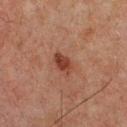Q: Is there a histopathology result?
A: total-body-photography surveillance lesion; no biopsy
Q: What are the patient's age and sex?
A: male, aged around 60
Q: What kind of image is this?
A: 15 mm crop, total-body photography
Q: Where on the body is the lesion?
A: the chest
Q: How large is the lesion?
A: ≈3 mm
Q: What did automated image analysis measure?
A: an eccentricity of roughly 0.75 and a shape-asymmetry score of about 0.15 (0 = symmetric); a border-irregularity index near 1.5/10, a within-lesion color-variation index near 3.5/10, and peripheral color asymmetry of about 1
Q: How was the tile lit?
A: cross-polarized